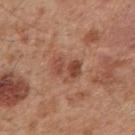Case summary:
– notes · no biopsy performed (imaged during a skin exam)
– illumination · white-light
– size · ≈3.5 mm
– TBP lesion metrics · a lesion color around L≈47 a*≈24 b*≈29 in CIELAB, about 10 CIELAB-L* units darker than the surrounding skin, and a normalized lesion–skin contrast near 7; radial color variation of about 2; lesion-presence confidence of about 100/100
– patient · male, in their mid- to late 40s
– location · the mid back
– acquisition · 15 mm crop, total-body photography